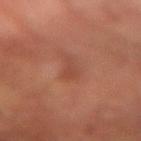No biopsy was performed on this lesion — it was imaged during a full skin examination and was not determined to be concerning.
On the left forearm.
The lesion's longest dimension is about 3 mm.
The lesion-visualizer software estimated a lesion color around L≈44 a*≈25 b*≈30 in CIELAB, about 6 CIELAB-L* units darker than the surrounding skin, and a normalized lesion–skin contrast near 5. It also reported a classifier nevus-likeness of about 0/100 and a detector confidence of about 100 out of 100 that the crop contains a lesion.
A male subject in their mid- to late 60s.
This image is a 15 mm lesion crop taken from a total-body photograph.
The tile uses cross-polarized illumination.